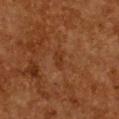No biopsy was performed on this lesion — it was imaged during a full skin examination and was not determined to be concerning.
A lesion tile, about 15 mm wide, cut from a 3D total-body photograph.
On the chest.
A female subject, aged approximately 50.